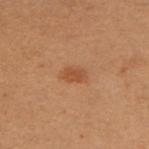No biopsy was performed on this lesion — it was imaged during a full skin examination and was not determined to be concerning.
Approximately 3 mm at its widest.
A region of skin cropped from a whole-body photographic capture, roughly 15 mm wide.
The lesion-visualizer software estimated a footprint of about 4.5 mm², an outline eccentricity of about 0.75 (0 = round, 1 = elongated), and a shape-asymmetry score of about 0.25 (0 = symmetric). The analysis additionally found an average lesion color of about L≈43 a*≈20 b*≈32 (CIELAB) and roughly 7 lightness units darker than nearby skin. It also reported border irregularity of about 2.5 on a 0–10 scale, a within-lesion color-variation index near 2/10, and peripheral color asymmetry of about 0.5.
The patient is a female aged approximately 50.
The lesion is on the left upper arm.
The tile uses cross-polarized illumination.